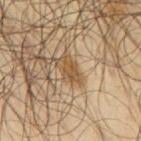Part of a total-body skin-imaging series; this lesion was reviewed on a skin check and was not flagged for biopsy. A male patient, aged around 65. Longest diameter approximately 3 mm. The lesion-visualizer software estimated a lesion color around L≈49 a*≈16 b*≈35 in CIELAB, about 10 CIELAB-L* units darker than the surrounding skin, and a normalized lesion–skin contrast near 8.5. A roughly 15 mm field-of-view crop from a total-body skin photograph. The lesion is located on the upper back. This is a cross-polarized tile.